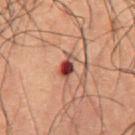The tile uses cross-polarized illumination. The lesion-visualizer software estimated an average lesion color of about L≈33 a*≈23 b*≈21 (CIELAB), about 14 CIELAB-L* units darker than the surrounding skin, and a normalized lesion–skin contrast near 12.5. A male patient in their 50s. A region of skin cropped from a whole-body photographic capture, roughly 15 mm wide. Located on the abdomen.